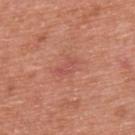notes = no biopsy performed (imaged during a skin exam); body site = the upper back; image source = 15 mm crop, total-body photography; patient = male, in their mid-60s.